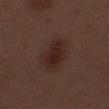  biopsy_status: not biopsied; imaged during a skin examination
  image:
    source: total-body photography crop
    field_of_view_mm: 15
  patient:
    sex: male
    age_approx: 70
  site: left thigh